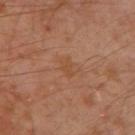* workup — imaged on a skin check; not biopsied
* anatomic site — the left arm
* imaging modality — total-body-photography crop, ~15 mm field of view
* patient — male, in their 30s
* image-analysis metrics — a footprint of about 3 mm², an eccentricity of roughly 0.9, and a shape-asymmetry score of about 0.4 (0 = symmetric); a mean CIELAB color near L≈46 a*≈21 b*≈33, roughly 5 lightness units darker than nearby skin, and a lesion-to-skin contrast of about 5 (normalized; higher = more distinct); internal color variation of about 1 on a 0–10 scale and radial color variation of about 0.5; a nevus-likeness score of about 0/100 and lesion-presence confidence of about 100/100
* diameter — ≈2.5 mm